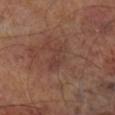Impression: Imaged during a routine full-body skin examination; the lesion was not biopsied and no histopathology is available. Image and clinical context: Captured under cross-polarized illumination. The lesion-visualizer software estimated a footprint of about 6 mm², an eccentricity of roughly 0.85, and a shape-asymmetry score of about 0.3 (0 = symmetric). It also reported a lesion–skin lightness drop of about 5 and a lesion-to-skin contrast of about 4.5 (normalized; higher = more distinct). It also reported an automated nevus-likeness rating near 0 out of 100 and a detector confidence of about 100 out of 100 that the crop contains a lesion. The recorded lesion diameter is about 4 mm. Cropped from a total-body skin-imaging series; the visible field is about 15 mm. A male subject, in their 70s. Located on the right lower leg.